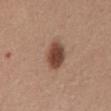{"image": {"source": "total-body photography crop", "field_of_view_mm": 15}, "lesion_size": {"long_diameter_mm_approx": 3.5}, "lighting": "white-light", "patient": {"sex": "male", "age_approx": 55}, "automated_metrics": {"area_mm2_approx": 8.5, "eccentricity": 0.65, "shape_asymmetry": 0.1, "cielab_L": 45, "cielab_a": 21, "cielab_b": 27, "vs_skin_darker_L": 15.0, "vs_skin_contrast_norm": 10.5, "border_irregularity_0_10": 1.0, "color_variation_0_10": 3.5, "nevus_likeness_0_100": 100, "lesion_detection_confidence_0_100": 100}, "site": "mid back"}